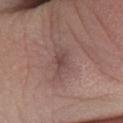<lesion>
<biopsy_status>not biopsied; imaged during a skin examination</biopsy_status>
<image>
  <source>total-body photography crop</source>
  <field_of_view_mm>15</field_of_view_mm>
</image>
<automated_metrics>
  <eccentricity>0.8</eccentricity>
  <shape_asymmetry>0.35</shape_asymmetry>
  <cielab_L>45</cielab_L>
  <cielab_a>19</cielab_a>
  <cielab_b>20</cielab_b>
  <vs_skin_darker_L>8.0</vs_skin_darker_L>
  <vs_skin_contrast_norm>6.0</vs_skin_contrast_norm>
  <color_variation_0_10>2.0</color_variation_0_10>
  <peripheral_color_asymmetry>0.5</peripheral_color_asymmetry>
</automated_metrics>
<lesion_size>
  <long_diameter_mm_approx>3.0</long_diameter_mm_approx>
</lesion_size>
<site>right lower leg</site>
<patient>
  <sex>female</sex>
  <age_approx>45</age_approx>
</patient>
<lighting>white-light</lighting>
</lesion>